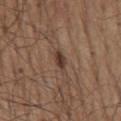notes = no biopsy performed (imaged during a skin exam); site = the mid back; acquisition = ~15 mm tile from a whole-body skin photo; patient = male, in their mid- to late 70s; lesion diameter = ≈3 mm.